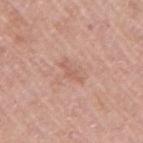{"biopsy_status": "not biopsied; imaged during a skin examination", "patient": {"sex": "female", "age_approx": 60}, "image": {"source": "total-body photography crop", "field_of_view_mm": 15}, "site": "right upper arm", "lighting": "white-light", "lesion_size": {"long_diameter_mm_approx": 3.0}}